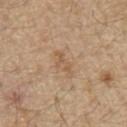Acquisition and patient details:
A lesion tile, about 15 mm wide, cut from a 3D total-body photograph. The patient is a male aged 68 to 72. Longest diameter approximately 3 mm. From the chest.